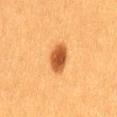Findings:
- notes: total-body-photography surveillance lesion; no biopsy
- image source: total-body-photography crop, ~15 mm field of view
- location: the lower back
- image-analysis metrics: a lesion area of about 7 mm², a shape eccentricity near 0.7, and a symmetry-axis asymmetry near 0.2
- illumination: cross-polarized illumination
- subject: female, roughly 40 years of age